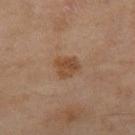Impression: The lesion was photographed on a routine skin check and not biopsied; there is no pathology result. Context: A female subject, about 60 years old. Located on the right thigh. A roughly 15 mm field-of-view crop from a total-body skin photograph. The lesion's longest dimension is about 3 mm.